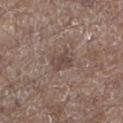Impression:
Recorded during total-body skin imaging; not selected for excision or biopsy.
Image and clinical context:
The lesion is located on the left lower leg. An algorithmic analysis of the crop reported a footprint of about 5.5 mm², a shape eccentricity near 0.6, and two-axis asymmetry of about 0.25. The analysis additionally found a lesion color around L≈45 a*≈15 b*≈21 in CIELAB. The analysis additionally found a border-irregularity rating of about 3/10, internal color variation of about 2 on a 0–10 scale, and a peripheral color-asymmetry measure near 0.5. The analysis additionally found an automated nevus-likeness rating near 0 out of 100 and lesion-presence confidence of about 100/100. A 15 mm close-up extracted from a 3D total-body photography capture. This is a white-light tile. The recorded lesion diameter is about 3 mm. A male subject, aged 53–57.